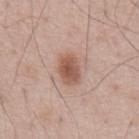- workup — imaged on a skin check; not biopsied
- patient — male, in their mid- to late 50s
- automated lesion analysis — a footprint of about 7.5 mm², a shape eccentricity near 0.7, and a shape-asymmetry score of about 0.15 (0 = symmetric); a nevus-likeness score of about 100/100
- size — ~3.5 mm (longest diameter)
- tile lighting — white-light illumination
- imaging modality — total-body-photography crop, ~15 mm field of view